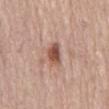Q: Is there a histopathology result?
A: imaged on a skin check; not biopsied
Q: What are the patient's age and sex?
A: male, aged 63 to 67
Q: How was this image acquired?
A: ~15 mm crop, total-body skin-cancer survey
Q: What is the anatomic site?
A: the back